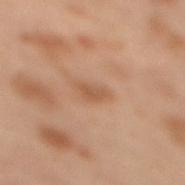- notes · total-body-photography surveillance lesion; no biopsy
- tile lighting · cross-polarized
- automated lesion analysis · a lesion area of about 3 mm², an eccentricity of roughly 0.8, and a symmetry-axis asymmetry near 0.4; a lesion–skin lightness drop of about 7; a border-irregularity index near 3.5/10, a within-lesion color-variation index near 1/10, and peripheral color asymmetry of about 0.5
- patient · female, in their mid- to late 50s
- body site · the upper back
- acquisition · 15 mm crop, total-body photography
- lesion diameter · ~2.5 mm (longest diameter)